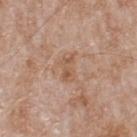Captured during whole-body skin photography for melanoma surveillance; the lesion was not biopsied.
Cropped from a total-body skin-imaging series; the visible field is about 15 mm.
Located on the back.
A male patient aged approximately 80.